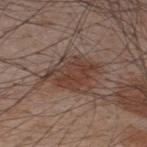image-analysis metrics: a footprint of about 17 mm²; a normalized border contrast of about 8
location: the upper back
imaging modality: total-body-photography crop, ~15 mm field of view
tile lighting: white-light
subject: male, roughly 35 years of age